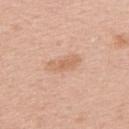location: the upper back | TBP lesion metrics: border irregularity of about 2.5 on a 0–10 scale, internal color variation of about 2 on a 0–10 scale, and radial color variation of about 0.5 | lighting: white-light | subject: male, about 65 years old | lesion diameter: about 4 mm | image source: total-body-photography crop, ~15 mm field of view.